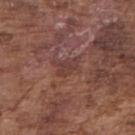<tbp_lesion>
  <lesion_size>
    <long_diameter_mm_approx>2.5</long_diameter_mm_approx>
  </lesion_size>
  <site>right upper arm</site>
  <patient>
    <sex>male</sex>
    <age_approx>75</age_approx>
  </patient>
  <image>
    <source>total-body photography crop</source>
    <field_of_view_mm>15</field_of_view_mm>
  </image>
</tbp_lesion>